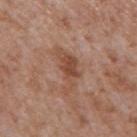Findings:
- biopsy status · imaged on a skin check; not biopsied
- size · ~5.5 mm (longest diameter)
- anatomic site · the mid back
- tile lighting · white-light illumination
- imaging modality · 15 mm crop, total-body photography
- automated lesion analysis · border irregularity of about 7 on a 0–10 scale, internal color variation of about 3 on a 0–10 scale, and radial color variation of about 1
- subject · male, in their mid- to late 60s